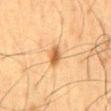Clinical impression:
The lesion was tiled from a total-body skin photograph and was not biopsied.
Clinical summary:
The patient is a male aged approximately 60. From the mid back. A lesion tile, about 15 mm wide, cut from a 3D total-body photograph. Automated image analysis of the tile measured an area of roughly 4 mm², a shape eccentricity near 0.8, and two-axis asymmetry of about 0.2. The analysis additionally found border irregularity of about 1.5 on a 0–10 scale, internal color variation of about 3.5 on a 0–10 scale, and radial color variation of about 1. This is a cross-polarized tile.